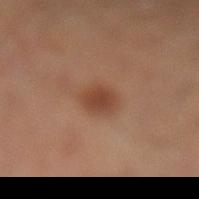Findings:
- biopsy status: catalogued during a skin exam; not biopsied
- image source: total-body-photography crop, ~15 mm field of view
- patient: male, aged 58 to 62
- body site: the left lower leg
- lesion diameter: ~3 mm (longest diameter)